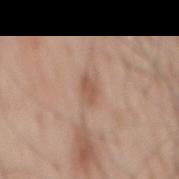Part of a total-body skin-imaging series; this lesion was reviewed on a skin check and was not flagged for biopsy.
Located on the mid back.
A 15 mm crop from a total-body photograph taken for skin-cancer surveillance.
The patient is a male aged approximately 70.